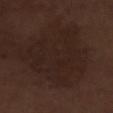<case>
  <automated_metrics>
    <area_mm2_approx>37.0</area_mm2_approx>
    <eccentricity>0.25</eccentricity>
    <shape_asymmetry>0.5</shape_asymmetry>
    <cielab_L>21</cielab_L>
    <cielab_a>14</cielab_a>
    <cielab_b>19</cielab_b>
    <vs_skin_darker_L>4.0</vs_skin_darker_L>
    <vs_skin_contrast_norm>5.0</vs_skin_contrast_norm>
    <border_irregularity_0_10>7.5</border_irregularity_0_10>
    <nevus_likeness_0_100>0</nevus_likeness_0_100>
    <lesion_detection_confidence_0_100>95</lesion_detection_confidence_0_100>
  </automated_metrics>
  <site>right lower leg</site>
  <patient>
    <sex>male</sex>
    <age_approx>70</age_approx>
  </patient>
  <image>
    <source>total-body photography crop</source>
    <field_of_view_mm>15</field_of_view_mm>
  </image>
  <lesion_size>
    <long_diameter_mm_approx>8.0</long_diameter_mm_approx>
  </lesion_size>
</case>